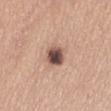{"biopsy_status": "not biopsied; imaged during a skin examination", "patient": {"sex": "female", "age_approx": 55}, "image": {"source": "total-body photography crop", "field_of_view_mm": 15}, "site": "abdomen", "lighting": "white-light", "lesion_size": {"long_diameter_mm_approx": 3.0}}